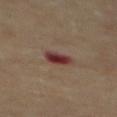| key | value |
|---|---|
| follow-up | no biopsy performed (imaged during a skin exam) |
| body site | the mid back |
| illumination | cross-polarized illumination |
| patient | male, approximately 85 years of age or older |
| acquisition | 15 mm crop, total-body photography |
| automated lesion analysis | an eccentricity of roughly 0.5 and a shape-asymmetry score of about 0.25 (0 = symmetric); an average lesion color of about L≈34 a*≈23 b*≈20 (CIELAB), roughly 13 lightness units darker than nearby skin, and a normalized border contrast of about 11.5 |